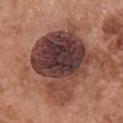Notes:
* size — ~9 mm (longest diameter)
* patient — female, approximately 65 years of age
* acquisition — 15 mm crop, total-body photography
* body site — the chest